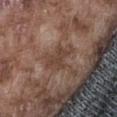Part of a total-body skin-imaging series; this lesion was reviewed on a skin check and was not flagged for biopsy. This is a white-light tile. On the abdomen. A 15 mm close-up extracted from a 3D total-body photography capture. The total-body-photography lesion software estimated an area of roughly 6.5 mm². The analysis additionally found a border-irregularity rating of about 5/10 and a color-variation rating of about 1.5/10. It also reported a classifier nevus-likeness of about 0/100. A male subject, approximately 75 years of age. Longest diameter approximately 4 mm.